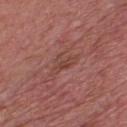Impression: The lesion was tiled from a total-body skin photograph and was not biopsied. Clinical summary: The tile uses white-light illumination. The recorded lesion diameter is about 4 mm. A male subject, about 70 years old. A roughly 15 mm field-of-view crop from a total-body skin photograph. Automated tile analysis of the lesion measured an area of roughly 4.5 mm², an outline eccentricity of about 0.9 (0 = round, 1 = elongated), and a symmetry-axis asymmetry near 0.6. The lesion is located on the chest.